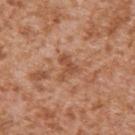Q: Was a biopsy performed?
A: catalogued during a skin exam; not biopsied
Q: What kind of image is this?
A: 15 mm crop, total-body photography
Q: Where on the body is the lesion?
A: the right upper arm
Q: Illumination type?
A: white-light illumination
Q: Who is the patient?
A: male, about 45 years old
Q: What did automated image analysis measure?
A: a lesion color around L≈51 a*≈24 b*≈34 in CIELAB, roughly 9 lightness units darker than nearby skin, and a lesion-to-skin contrast of about 6.5 (normalized; higher = more distinct); a border-irregularity rating of about 7/10, a color-variation rating of about 1.5/10, and radial color variation of about 0.5
Q: What is the lesion's diameter?
A: ≈3.5 mm